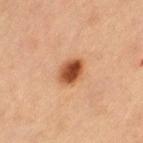This lesion was catalogued during total-body skin photography and was not selected for biopsy.
Cropped from a total-body skin-imaging series; the visible field is about 15 mm.
A female subject, in their 70s.
From the left thigh.